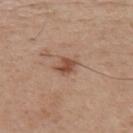workup — total-body-photography surveillance lesion; no biopsy
image — 15 mm crop, total-body photography
patient — male, in their mid-60s
body site — the back
size — about 2.5 mm
image-analysis metrics — a footprint of about 4 mm² and a symmetry-axis asymmetry near 0.3; border irregularity of about 2.5 on a 0–10 scale, a within-lesion color-variation index near 2.5/10, and radial color variation of about 0.5